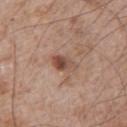A 15 mm close-up extracted from a 3D total-body photography capture.
A male subject, in their mid- to late 60s.
The lesion is on the left upper arm.